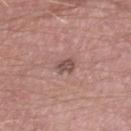Notes:
* notes: imaged on a skin check; not biopsied
* body site: the right thigh
* diameter: about 2 mm
* acquisition: ~15 mm tile from a whole-body skin photo
* patient: male, aged around 40